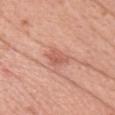  biopsy_status: not biopsied; imaged during a skin examination
  automated_metrics:
    area_mm2_approx: 5.0
    eccentricity: 0.65
    shape_asymmetry: 0.3
    border_irregularity_0_10: 3.0
    color_variation_0_10: 2.0
    peripheral_color_asymmetry: 1.0
  lighting: white-light
  patient:
    sex: female
    age_approx: 60
  lesion_size:
    long_diameter_mm_approx: 3.0
  image:
    source: total-body photography crop
    field_of_view_mm: 15
  site: head or neck